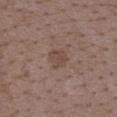Findings:
- notes — total-body-photography surveillance lesion; no biopsy
- lighting — white-light illumination
- size — about 3 mm
- subject — female, aged 33–37
- acquisition — ~15 mm crop, total-body skin-cancer survey
- location — the upper back